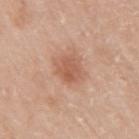Captured during whole-body skin photography for melanoma surveillance; the lesion was not biopsied.
An algorithmic analysis of the crop reported an eccentricity of roughly 0.15 and a symmetry-axis asymmetry near 0.25.
Cropped from a whole-body photographic skin survey; the tile spans about 15 mm.
A female patient, roughly 55 years of age.
The recorded lesion diameter is about 3 mm.
The lesion is on the arm.